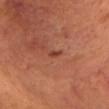notes = imaged on a skin check; not biopsied
tile lighting = cross-polarized illumination
subject = male, roughly 50 years of age
lesion diameter = ~1.5 mm (longest diameter)
image = ~15 mm tile from a whole-body skin photo
anatomic site = the head or neck